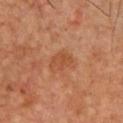- notes · imaged on a skin check; not biopsied
- tile lighting · cross-polarized illumination
- anatomic site · the chest
- acquisition · ~15 mm crop, total-body skin-cancer survey
- patient · male, roughly 50 years of age
- lesion diameter · ≈2.5 mm